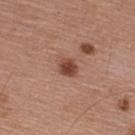patient:
  sex: male
  age_approx: 55
image:
  source: total-body photography crop
  field_of_view_mm: 15
automated_metrics:
  cielab_L: 46
  cielab_a: 23
  cielab_b: 29
  vs_skin_darker_L: 12.0
  vs_skin_contrast_norm: 9.0
  nevus_likeness_0_100: 95
  lesion_detection_confidence_0_100: 100
site: upper back
lesion_size:
  long_diameter_mm_approx: 2.5
lighting: white-light